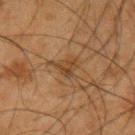Assessment: Imaged during a routine full-body skin examination; the lesion was not biopsied and no histopathology is available. Background: The patient is a male aged approximately 65. Captured under cross-polarized illumination. On the left upper arm. A 15 mm close-up extracted from a 3D total-body photography capture.